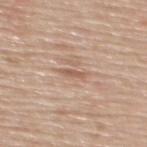Acquisition and patient details:
A 15 mm crop from a total-body photograph taken for skin-cancer surveillance. Approximately 2.5 mm at its widest. From the upper back. The subject is a male roughly 60 years of age.